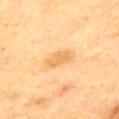workup = catalogued during a skin exam; not biopsied
subject = male, aged around 55
tile lighting = cross-polarized illumination
acquisition = ~15 mm tile from a whole-body skin photo
anatomic site = the upper back